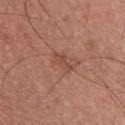• notes · catalogued during a skin exam; not biopsied
• image · total-body-photography crop, ~15 mm field of view
• subject · male, in their mid-30s
• tile lighting · white-light illumination
• image-analysis metrics · a mean CIELAB color near L≈48 a*≈23 b*≈28, a lesion–skin lightness drop of about 7, and a normalized border contrast of about 5.5; border irregularity of about 4.5 on a 0–10 scale, a within-lesion color-variation index near 1/10, and a peripheral color-asymmetry measure near 0.5; a nevus-likeness score of about 0/100 and a lesion-detection confidence of about 100/100
• site · the chest